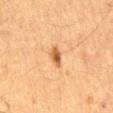Impression:
Recorded during total-body skin imaging; not selected for excision or biopsy.
Context:
The total-body-photography lesion software estimated a mean CIELAB color near L≈50 a*≈21 b*≈36, roughly 12 lightness units darker than nearby skin, and a normalized lesion–skin contrast near 8.5. Longest diameter approximately 2.5 mm. The lesion is on the mid back. A male subject aged around 65. Imaged with cross-polarized lighting. A 15 mm close-up extracted from a 3D total-body photography capture.